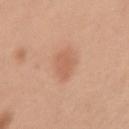Q: Was this lesion biopsied?
A: no biopsy performed (imaged during a skin exam)
Q: How large is the lesion?
A: ≈3.5 mm
Q: What is the imaging modality?
A: ~15 mm tile from a whole-body skin photo
Q: Who is the patient?
A: female, aged around 40
Q: What lighting was used for the tile?
A: white-light illumination
Q: Where on the body is the lesion?
A: the arm